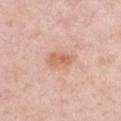The lesion was photographed on a routine skin check and not biopsied; there is no pathology result. Imaged with white-light lighting. About 3.5 mm across. From the right upper arm. A 15 mm close-up extracted from a 3D total-body photography capture. A male subject, about 50 years old.